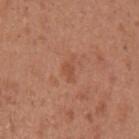The lesion was tiled from a total-body skin photograph and was not biopsied. Located on the left upper arm. Imaged with white-light lighting. The lesion-visualizer software estimated an area of roughly 3 mm², an eccentricity of roughly 0.85, and a symmetry-axis asymmetry near 0.5. And it measured a mean CIELAB color near L≈50 a*≈25 b*≈33. And it measured a detector confidence of about 100 out of 100 that the crop contains a lesion. A 15 mm crop from a total-body photograph taken for skin-cancer surveillance. The lesion's longest dimension is about 2.5 mm. A female subject, aged around 30.